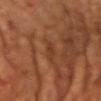Assessment: This lesion was catalogued during total-body skin photography and was not selected for biopsy. Context: This is a cross-polarized tile. A male patient in their 60s. The lesion is located on the back. A 15 mm close-up extracted from a 3D total-body photography capture. The recorded lesion diameter is about 3 mm.